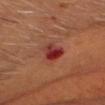{"biopsy_status": "not biopsied; imaged during a skin examination", "patient": {"sex": "male", "age_approx": 60}, "lighting": "cross-polarized", "image": {"source": "total-body photography crop", "field_of_view_mm": 15}, "lesion_size": {"long_diameter_mm_approx": 3.5}, "site": "head or neck", "automated_metrics": {"cielab_L": 34, "cielab_a": 32, "cielab_b": 26, "vs_skin_darker_L": 11.0, "border_irregularity_0_10": 2.5, "color_variation_0_10": 9.0, "peripheral_color_asymmetry": 3.0, "nevus_likeness_0_100": 0, "lesion_detection_confidence_0_100": 100}}